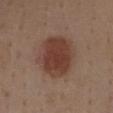Recorded during total-body skin imaging; not selected for excision or biopsy.
Cropped from a whole-body photographic skin survey; the tile spans about 15 mm.
A female patient, aged 38–42.
On the chest.
This is a white-light tile.